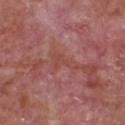Case summary:
* follow-up · total-body-photography surveillance lesion; no biopsy
* subject · male, aged 63–67
* lesion size · about 4 mm
* imaging modality · ~15 mm crop, total-body skin-cancer survey
* location · the front of the torso